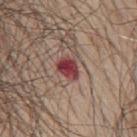The lesion was photographed on a routine skin check and not biopsied; there is no pathology result. On the back. The total-body-photography lesion software estimated an area of roughly 5 mm², an outline eccentricity of about 0.65 (0 = round, 1 = elongated), and a symmetry-axis asymmetry near 0.25. And it measured about 15 CIELAB-L* units darker than the surrounding skin and a normalized border contrast of about 11.5. A male patient roughly 70 years of age. The recorded lesion diameter is about 3 mm. Cropped from a total-body skin-imaging series; the visible field is about 15 mm.